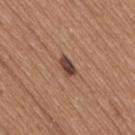{
  "biopsy_status": "not biopsied; imaged during a skin examination",
  "image": {
    "source": "total-body photography crop",
    "field_of_view_mm": 15
  },
  "patient": {
    "sex": "female",
    "age_approx": 35
  },
  "lesion_size": {
    "long_diameter_mm_approx": 2.5
  },
  "lighting": "white-light",
  "site": "right thigh"
}